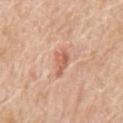diameter: about 3.5 mm; patient: male, aged 63–67; imaging modality: total-body-photography crop, ~15 mm field of view; location: the front of the torso; illumination: white-light illumination.